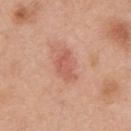notes — no biopsy performed (imaged during a skin exam)
illumination — white-light
diameter — ≈3.5 mm
image source — ~15 mm crop, total-body skin-cancer survey
site — the chest
subject — male, aged around 70